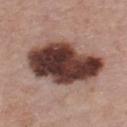notes — no biopsy performed (imaged during a skin exam) | tile lighting — white-light illumination | lesion size — ~9.5 mm (longest diameter) | patient — male, about 70 years old | body site — the front of the torso | image source — 15 mm crop, total-body photography.